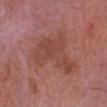Clinical impression:
This lesion was catalogued during total-body skin photography and was not selected for biopsy.
Context:
The lesion is on the left lower leg. This image is a 15 mm lesion crop taken from a total-body photograph. Longest diameter approximately 7.5 mm. A male subject, aged approximately 85 or older. Captured under white-light illumination.